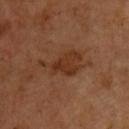Clinical summary:
This image is a 15 mm lesion crop taken from a total-body photograph. The lesion is on the chest. Imaged with cross-polarized lighting. A male patient, in their 50s. Automated image analysis of the tile measured a footprint of about 10 mm² and an outline eccentricity of about 0.8 (0 = round, 1 = elongated). It also reported a lesion color around L≈33 a*≈22 b*≈31 in CIELAB and roughly 7 lightness units darker than nearby skin. And it measured a border-irregularity rating of about 5.5/10, a within-lesion color-variation index near 3/10, and a peripheral color-asymmetry measure near 1. The analysis additionally found a classifier nevus-likeness of about 10/100.